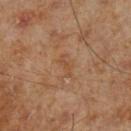Impression: The lesion was tiled from a total-body skin photograph and was not biopsied. Clinical summary: Located on the left lower leg. An algorithmic analysis of the crop reported a border-irregularity index near 5/10, internal color variation of about 0 on a 0–10 scale, and radial color variation of about 0. About 3 mm across. This is a cross-polarized tile. A close-up tile cropped from a whole-body skin photograph, about 15 mm across. The patient is a male aged around 70.